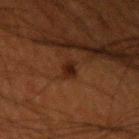biopsy status: total-body-photography surveillance lesion; no biopsy
tile lighting: cross-polarized
image: total-body-photography crop, ~15 mm field of view
image-analysis metrics: a lesion area of about 3.5 mm², an outline eccentricity of about 0.7 (0 = round, 1 = elongated), and two-axis asymmetry of about 0.3; a mean CIELAB color near L≈18 a*≈17 b*≈22 and a lesion-to-skin contrast of about 8.5 (normalized; higher = more distinct); border irregularity of about 3 on a 0–10 scale, internal color variation of about 2 on a 0–10 scale, and a peripheral color-asymmetry measure near 0.5; a classifier nevus-likeness of about 50/100 and a lesion-detection confidence of about 100/100
body site: the left upper arm
patient: male, approximately 50 years of age